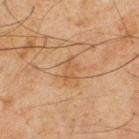No biopsy was performed on this lesion — it was imaged during a full skin examination and was not determined to be concerning. On the back. A male patient, in their mid- to late 40s. The recorded lesion diameter is about 3 mm. Imaged with cross-polarized lighting. A lesion tile, about 15 mm wide, cut from a 3D total-body photograph. The total-body-photography lesion software estimated a mean CIELAB color near L≈45 a*≈17 b*≈33, a lesion–skin lightness drop of about 5, and a normalized border contrast of about 5. The software also gave a border-irregularity rating of about 4/10. And it measured a classifier nevus-likeness of about 0/100.